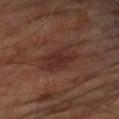<tbp_lesion>
  <image>
    <source>total-body photography crop</source>
    <field_of_view_mm>15</field_of_view_mm>
  </image>
  <patient>
    <sex>male</sex>
    <age_approx>65</age_approx>
  </patient>
  <site>right upper arm</site>
  <lesion_size>
    <long_diameter_mm_approx>5.0</long_diameter_mm_approx>
  </lesion_size>
  <lighting>cross-polarized</lighting>
</tbp_lesion>